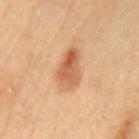Clinical impression:
The lesion was tiled from a total-body skin photograph and was not biopsied.
Image and clinical context:
The tile uses cross-polarized illumination. The total-body-photography lesion software estimated a border-irregularity rating of about 2.5/10, a color-variation rating of about 6.5/10, and peripheral color asymmetry of about 2.5. The recorded lesion diameter is about 4.5 mm. A male patient, aged 83 or over. Cropped from a total-body skin-imaging series; the visible field is about 15 mm. Located on the mid back.